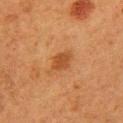Clinical impression: Imaged during a routine full-body skin examination; the lesion was not biopsied and no histopathology is available. Image and clinical context: Measured at roughly 3 mm in maximum diameter. The lesion is on the chest. The subject is a female approximately 50 years of age. Captured under cross-polarized illumination. Cropped from a whole-body photographic skin survey; the tile spans about 15 mm.